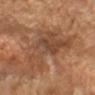Assessment: The lesion was photographed on a routine skin check and not biopsied; there is no pathology result. Context: A 15 mm close-up tile from a total-body photography series done for melanoma screening. The tile uses cross-polarized illumination. The lesion is on the left forearm. The subject is a female aged approximately 70.